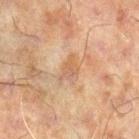Q: Was this lesion biopsied?
A: imaged on a skin check; not biopsied
Q: Lesion location?
A: the leg
Q: Lesion size?
A: ~3 mm (longest diameter)
Q: What did automated image analysis measure?
A: a footprint of about 4 mm² and an eccentricity of roughly 0.75; border irregularity of about 4 on a 0–10 scale and internal color variation of about 3 on a 0–10 scale
Q: Patient demographics?
A: male, roughly 60 years of age
Q: What kind of image is this?
A: 15 mm crop, total-body photography
Q: How was the tile lit?
A: cross-polarized illumination